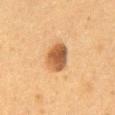notes=catalogued during a skin exam; not biopsied | site=the front of the torso | imaging modality=15 mm crop, total-body photography | lesion size=~3.5 mm (longest diameter) | illumination=cross-polarized | subject=female, in their 50s.